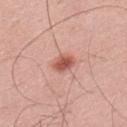Assessment: Part of a total-body skin-imaging series; this lesion was reviewed on a skin check and was not flagged for biopsy. Background: A male patient approximately 35 years of age. An algorithmic analysis of the crop reported an area of roughly 5 mm², an eccentricity of roughly 0.75, and two-axis asymmetry of about 0.2. It also reported a mean CIELAB color near L≈57 a*≈26 b*≈29. Longest diameter approximately 3 mm. On the left upper arm. This is a white-light tile. A close-up tile cropped from a whole-body skin photograph, about 15 mm across.